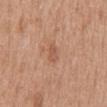follow-up: catalogued during a skin exam; not biopsied
location: the mid back
illumination: white-light
subject: female, roughly 40 years of age
size: ≈2.5 mm
imaging modality: ~15 mm crop, total-body skin-cancer survey